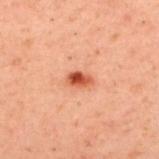Part of a total-body skin-imaging series; this lesion was reviewed on a skin check and was not flagged for biopsy. This is a cross-polarized tile. On the upper back. A male subject, about 40 years old. A region of skin cropped from a whole-body photographic capture, roughly 15 mm wide. About 2.5 mm across.